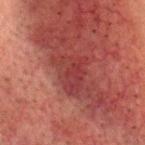The lesion was tiled from a total-body skin photograph and was not biopsied. An algorithmic analysis of the crop reported a footprint of about 10 mm² and a symmetry-axis asymmetry near 0.4. It also reported a mean CIELAB color near L≈31 a*≈26 b*≈20, roughly 6 lightness units darker than nearby skin, and a normalized lesion–skin contrast near 6. It also reported border irregularity of about 5 on a 0–10 scale, a within-lesion color-variation index near 3.5/10, and peripheral color asymmetry of about 1. It also reported a nevus-likeness score of about 0/100 and a detector confidence of about 85 out of 100 that the crop contains a lesion. Imaged with cross-polarized lighting. A male patient, aged 78 to 82. Approximately 4.5 mm at its widest. From the head or neck. Cropped from a total-body skin-imaging series; the visible field is about 15 mm.